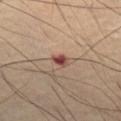Imaged during a routine full-body skin examination; the lesion was not biopsied and no histopathology is available. Cropped from a total-body skin-imaging series; the visible field is about 15 mm. A male subject, aged 53 to 57. The lesion is located on the abdomen.